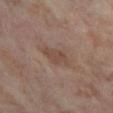Notes:
* biopsy status — imaged on a skin check; not biopsied
* patient — female, approximately 55 years of age
* acquisition — 15 mm crop, total-body photography
* body site — the leg
* illumination — cross-polarized illumination
* TBP lesion metrics — a lesion area of about 6 mm², a shape eccentricity near 0.8, and a symmetry-axis asymmetry near 0.2; a mean CIELAB color near L≈46 a*≈16 b*≈25